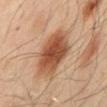Recorded during total-body skin imaging; not selected for excision or biopsy. Approximately 6.5 mm at its widest. The total-body-photography lesion software estimated an average lesion color of about L≈48 a*≈22 b*≈32 (CIELAB) and about 14 CIELAB-L* units darker than the surrounding skin. A male patient in their mid- to late 60s. On the mid back. The tile uses cross-polarized illumination. A region of skin cropped from a whole-body photographic capture, roughly 15 mm wide.